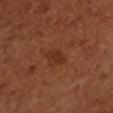Part of a total-body skin-imaging series; this lesion was reviewed on a skin check and was not flagged for biopsy. From the left forearm. The tile uses cross-polarized illumination. The lesion-visualizer software estimated an eccentricity of roughly 0.85 and a shape-asymmetry score of about 0.25 (0 = symmetric). And it measured an average lesion color of about L≈32 a*≈25 b*≈32 (CIELAB), a lesion–skin lightness drop of about 6, and a normalized border contrast of about 6. And it measured a border-irregularity index near 2.5/10 and a peripheral color-asymmetry measure near 0.5. The software also gave a classifier nevus-likeness of about 0/100. A female subject roughly 55 years of age. The recorded lesion diameter is about 2.5 mm. A lesion tile, about 15 mm wide, cut from a 3D total-body photograph.